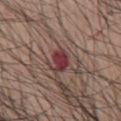workup: total-body-photography surveillance lesion; no biopsy | body site: the back | lighting: white-light illumination | subject: male, aged around 70 | acquisition: total-body-photography crop, ~15 mm field of view.